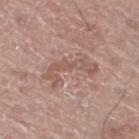Imaged during a routine full-body skin examination; the lesion was not biopsied and no histopathology is available.
About 6 mm across.
This is a white-light tile.
A close-up tile cropped from a whole-body skin photograph, about 15 mm across.
Located on the right thigh.
The subject is a male aged around 75.
Automated tile analysis of the lesion measured a lesion color around L≈55 a*≈20 b*≈25 in CIELAB and a lesion–skin lightness drop of about 8.